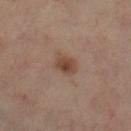diameter = ~3 mm (longest diameter)
tile lighting = cross-polarized illumination
acquisition = total-body-photography crop, ~15 mm field of view
subject = female, in their mid-50s
anatomic site = the left leg